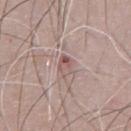The lesion was tiled from a total-body skin photograph and was not biopsied. A male subject, about 65 years old. The lesion is on the abdomen. About 2.5 mm across. A lesion tile, about 15 mm wide, cut from a 3D total-body photograph.